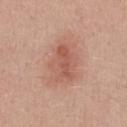Findings:
– follow-up · no biopsy performed (imaged during a skin exam)
– imaging modality · total-body-photography crop, ~15 mm field of view
– location · the chest
– subject · male, aged approximately 30